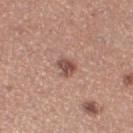workup = imaged on a skin check; not biopsied.